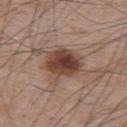Q: Is there a histopathology result?
A: imaged on a skin check; not biopsied
Q: Patient demographics?
A: male, aged approximately 65
Q: What is the anatomic site?
A: the upper back
Q: What is the imaging modality?
A: ~15 mm tile from a whole-body skin photo